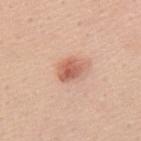Imaged during a routine full-body skin examination; the lesion was not biopsied and no histopathology is available. The lesion is on the mid back. Longest diameter approximately 3 mm. A female subject, aged approximately 45. A close-up tile cropped from a whole-body skin photograph, about 15 mm across. The lesion-visualizer software estimated an eccentricity of roughly 0.65 and two-axis asymmetry of about 0.25. The software also gave a lesion color around L≈60 a*≈26 b*≈31 in CIELAB, a lesion–skin lightness drop of about 12, and a lesion-to-skin contrast of about 8 (normalized; higher = more distinct). It also reported an automated nevus-likeness rating near 100 out of 100 and a lesion-detection confidence of about 100/100.